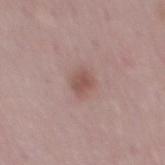biopsy status — imaged on a skin check; not biopsied | image-analysis metrics — an eccentricity of roughly 0.65; a mean CIELAB color near L≈52 a*≈20 b*≈23 and a normalized lesion–skin contrast near 7; a border-irregularity rating of about 2/10, internal color variation of about 2 on a 0–10 scale, and a peripheral color-asymmetry measure near 0.5; an automated nevus-likeness rating near 70 out of 100 and a detector confidence of about 100 out of 100 that the crop contains a lesion | location — the mid back | tile lighting — white-light | patient — male, aged 48–52 | imaging modality — total-body-photography crop, ~15 mm field of view.